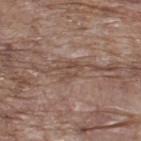{"image": {"source": "total-body photography crop", "field_of_view_mm": 15}, "patient": {"sex": "male", "age_approx": 70}, "site": "upper back", "lesion_size": {"long_diameter_mm_approx": 3.0}, "lighting": "white-light"}